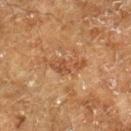Impression: Part of a total-body skin-imaging series; this lesion was reviewed on a skin check and was not flagged for biopsy. Acquisition and patient details: The recorded lesion diameter is about 5.5 mm. A 15 mm close-up extracted from a 3D total-body photography capture. A male patient, in their 60s. The lesion-visualizer software estimated a footprint of about 7 mm² and two-axis asymmetry of about 0.4. The analysis additionally found a border-irregularity rating of about 5.5/10, a within-lesion color-variation index near 3/10, and a peripheral color-asymmetry measure near 1. And it measured a nevus-likeness score of about 0/100 and a detector confidence of about 90 out of 100 that the crop contains a lesion. The lesion is located on the right lower leg.